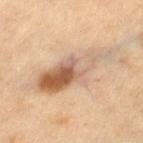No biopsy was performed on this lesion — it was imaged during a full skin examination and was not determined to be concerning. Captured under cross-polarized illumination. A female patient, in their mid-50s. The lesion-visualizer software estimated an area of roughly 24 mm² and an outline eccentricity of about 0.9 (0 = round, 1 = elongated). And it measured a nevus-likeness score of about 95/100 and lesion-presence confidence of about 100/100. The recorded lesion diameter is about 9.5 mm. This image is a 15 mm lesion crop taken from a total-body photograph. Located on the right thigh.